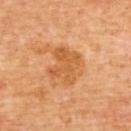{"biopsy_status": "not biopsied; imaged during a skin examination", "patient": {"sex": "male", "age_approx": 60}, "site": "back", "image": {"source": "total-body photography crop", "field_of_view_mm": 15}, "automated_metrics": {"area_mm2_approx": 11.0, "eccentricity": 0.5, "shape_asymmetry": 0.2, "lesion_detection_confidence_0_100": 100}, "lighting": "cross-polarized"}